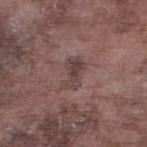| key | value |
|---|---|
| notes | imaged on a skin check; not biopsied |
| location | the left lower leg |
| imaging modality | ~15 mm crop, total-body skin-cancer survey |
| patient | male, aged approximately 75 |